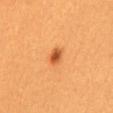location = the mid back | TBP lesion metrics = an automated nevus-likeness rating near 100 out of 100 and a lesion-detection confidence of about 100/100 | subject = female, aged approximately 30 | lighting = cross-polarized | imaging modality = total-body-photography crop, ~15 mm field of view | size = about 2.5 mm.